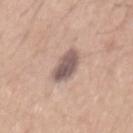Impression:
The lesion was tiled from a total-body skin photograph and was not biopsied.
Image and clinical context:
Automated image analysis of the tile measured a footprint of about 9 mm², an outline eccentricity of about 0.8 (0 = round, 1 = elongated), and a shape-asymmetry score of about 0.15 (0 = symmetric). It also reported a mean CIELAB color near L≈56 a*≈16 b*≈21 and roughly 14 lightness units darker than nearby skin. The analysis additionally found a color-variation rating of about 4.5/10. It also reported a nevus-likeness score of about 30/100 and lesion-presence confidence of about 100/100. This is a white-light tile. A 15 mm close-up extracted from a 3D total-body photography capture. On the mid back. The recorded lesion diameter is about 4.5 mm. The subject is a male approximately 30 years of age.